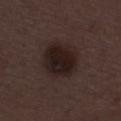This lesion was catalogued during total-body skin photography and was not selected for biopsy. Measured at roughly 4.5 mm in maximum diameter. The patient is a female aged approximately 50. A 15 mm close-up tile from a total-body photography series done for melanoma screening. Imaged with white-light lighting. From the left lower leg.